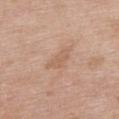The lesion was tiled from a total-body skin photograph and was not biopsied. A lesion tile, about 15 mm wide, cut from a 3D total-body photograph. Automated tile analysis of the lesion measured a lesion color around L≈60 a*≈19 b*≈31 in CIELAB and a lesion-to-skin contrast of about 5 (normalized; higher = more distinct). The software also gave an automated nevus-likeness rating near 0 out of 100 and a detector confidence of about 100 out of 100 that the crop contains a lesion. On the back. This is a white-light tile. Approximately 3.5 mm at its widest. The patient is a female aged around 50.